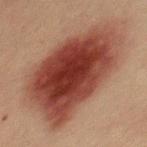The tile uses cross-polarized illumination.
A male patient, aged approximately 40.
A 15 mm close-up tile from a total-body photography series done for melanoma screening.
Located on the chest.
The total-body-photography lesion software estimated a footprint of about 65 mm², a shape eccentricity near 0.8, and two-axis asymmetry of about 0.2. And it measured a mean CIELAB color near L≈34 a*≈22 b*≈24, roughly 14 lightness units darker than nearby skin, and a normalized border contrast of about 12.5. And it measured a classifier nevus-likeness of about 100/100 and lesion-presence confidence of about 100/100.
About 12 mm across.